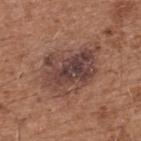follow-up: imaged on a skin check; not biopsied | diameter: ≈7.5 mm | anatomic site: the back | TBP lesion metrics: a lesion-to-skin contrast of about 9 (normalized; higher = more distinct); a classifier nevus-likeness of about 15/100 and a lesion-detection confidence of about 100/100 | patient: male, approximately 75 years of age | image source: ~15 mm crop, total-body skin-cancer survey | lighting: white-light.